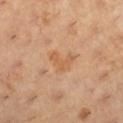This lesion was catalogued during total-body skin photography and was not selected for biopsy.
Cropped from a total-body skin-imaging series; the visible field is about 15 mm.
The lesion-visualizer software estimated a footprint of about 5 mm², a shape eccentricity near 0.7, and a shape-asymmetry score of about 0.7 (0 = symmetric). The analysis additionally found an average lesion color of about L≈59 a*≈22 b*≈38 (CIELAB) and about 7 CIELAB-L* units darker than the surrounding skin. It also reported a nevus-likeness score of about 0/100 and lesion-presence confidence of about 100/100.
A female subject, in their 30s.
Captured under cross-polarized illumination.
From the right lower leg.